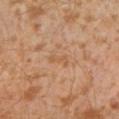biopsy status=no biopsy performed (imaged during a skin exam) | lesion size=about 2.5 mm | image source=~15 mm crop, total-body skin-cancer survey | lighting=cross-polarized | site=the left lower leg | patient=male, aged 28–32.